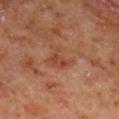- follow-up — catalogued during a skin exam; not biopsied
- body site — the leg
- image — ~15 mm tile from a whole-body skin photo
- patient — male, approximately 60 years of age